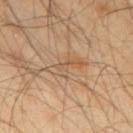Recorded during total-body skin imaging; not selected for excision or biopsy.
Automated image analysis of the tile measured a lesion area of about 6 mm², an outline eccentricity of about 0.95 (0 = round, 1 = elongated), and a shape-asymmetry score of about 0.5 (0 = symmetric). It also reported roughly 7 lightness units darker than nearby skin and a normalized lesion–skin contrast near 5. It also reported a nevus-likeness score of about 0/100 and lesion-presence confidence of about 75/100.
From the upper back.
A male patient, in their 40s.
This image is a 15 mm lesion crop taken from a total-body photograph.
This is a cross-polarized tile.